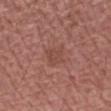Assessment: The lesion was tiled from a total-body skin photograph and was not biopsied. Clinical summary: The recorded lesion diameter is about 2.5 mm. From the left forearm. Cropped from a total-body skin-imaging series; the visible field is about 15 mm. Captured under white-light illumination. A male subject, aged approximately 70.